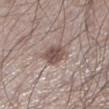Impression: No biopsy was performed on this lesion — it was imaged during a full skin examination and was not determined to be concerning. Acquisition and patient details: The subject is a male about 60 years old. The tile uses white-light illumination. Located on the left lower leg. A close-up tile cropped from a whole-body skin photograph, about 15 mm across. Longest diameter approximately 3.5 mm.